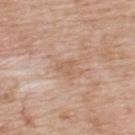notes=total-body-photography surveillance lesion; no biopsy | patient=male, aged 58–62 | automated metrics=border irregularity of about 5.5 on a 0–10 scale and a within-lesion color-variation index near 1.5/10 | location=the upper back | lighting=white-light | lesion size=≈2.5 mm | acquisition=~15 mm crop, total-body skin-cancer survey.